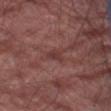Clinical impression:
The lesion was tiled from a total-body skin photograph and was not biopsied.
Clinical summary:
This image is a 15 mm lesion crop taken from a total-body photograph. A male subject, approximately 80 years of age. The lesion is located on the right leg.